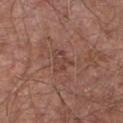follow-up: no biopsy performed (imaged during a skin exam)
lighting: white-light
subject: male, in their mid-60s
automated lesion analysis: a mean CIELAB color near L≈43 a*≈21 b*≈25, about 6 CIELAB-L* units darker than the surrounding skin, and a normalized border contrast of about 5; border irregularity of about 4 on a 0–10 scale, a color-variation rating of about 2.5/10, and radial color variation of about 1
site: the chest
image source: ~15 mm tile from a whole-body skin photo